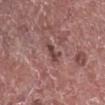The lesion was photographed on a routine skin check and not biopsied; there is no pathology result. Longest diameter approximately 3 mm. Imaged with white-light lighting. From the right lower leg. Cropped from a whole-body photographic skin survey; the tile spans about 15 mm. A male subject, aged approximately 75.